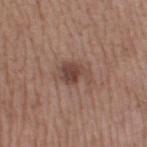* notes · imaged on a skin check; not biopsied
* image · ~15 mm crop, total-body skin-cancer survey
* lesion diameter · ≈4 mm
* automated lesion analysis · a footprint of about 7.5 mm² and an outline eccentricity of about 0.75 (0 = round, 1 = elongated); a lesion color around L≈44 a*≈18 b*≈23 in CIELAB and a normalized border contrast of about 8
* site · the right thigh
* tile lighting · white-light
* subject · female, roughly 55 years of age